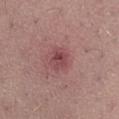Context:
The lesion is located on the right thigh. Measured at roughly 3 mm in maximum diameter. Captured under white-light illumination. Automated tile analysis of the lesion measured an area of roughly 5.5 mm², a shape eccentricity near 0.65, and a shape-asymmetry score of about 0.25 (0 = symmetric). And it measured a lesion color around L≈46 a*≈26 b*≈18 in CIELAB. And it measured a border-irregularity rating of about 2.5/10, a within-lesion color-variation index near 5/10, and peripheral color asymmetry of about 2. And it measured a lesion-detection confidence of about 100/100. A close-up tile cropped from a whole-body skin photograph, about 15 mm across. The subject is a male aged around 40.